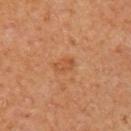| field | value |
|---|---|
| biopsy status | total-body-photography surveillance lesion; no biopsy |
| image | 15 mm crop, total-body photography |
| patient | female |
| illumination | cross-polarized |
| body site | the upper back |
| TBP lesion metrics | a border-irregularity rating of about 3/10 and peripheral color asymmetry of about 0; a nevus-likeness score of about 20/100 and a detector confidence of about 100 out of 100 that the crop contains a lesion |